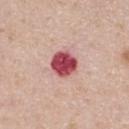  biopsy_status: not biopsied; imaged during a skin examination
  lesion_size:
    long_diameter_mm_approx: 3.0
  automated_metrics:
    area_mm2_approx: 8.0
    eccentricity: 0.25
    shape_asymmetry: 0.15
    nevus_likeness_0_100: 0
    lesion_detection_confidence_0_100: 100
  image:
    source: total-body photography crop
    field_of_view_mm: 15
  site: upper back
  patient:
    sex: male
    age_approx: 40